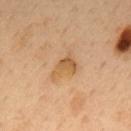The lesion was tiled from a total-body skin photograph and was not biopsied. Automated image analysis of the tile measured an area of roughly 5.5 mm² and an eccentricity of roughly 0.75. It also reported a border-irregularity rating of about 6/10 and radial color variation of about 1. And it measured an automated nevus-likeness rating near 0 out of 100 and lesion-presence confidence of about 100/100. The subject is a male in their 50s. On the mid back. Captured under cross-polarized illumination. Cropped from a total-body skin-imaging series; the visible field is about 15 mm.